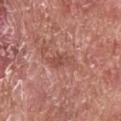The subject is a male aged 63–67. The recorded lesion diameter is about 3.5 mm. Automated tile analysis of the lesion measured an average lesion color of about L≈50 a*≈26 b*≈27 (CIELAB), a lesion–skin lightness drop of about 8, and a normalized lesion–skin contrast near 6. And it measured a border-irregularity index near 3.5/10, a within-lesion color-variation index near 3/10, and peripheral color asymmetry of about 1. And it measured a nevus-likeness score of about 0/100 and a lesion-detection confidence of about 100/100. Located on the back. A close-up tile cropped from a whole-body skin photograph, about 15 mm across. Imaged with white-light lighting.